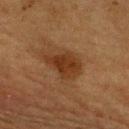<record>
  <biopsy_status>not biopsied; imaged during a skin examination</biopsy_status>
  <automated_metrics>
    <vs_skin_darker_L>8.0</vs_skin_darker_L>
    <vs_skin_contrast_norm>8.5</vs_skin_contrast_norm>
    <nevus_likeness_0_100>50</nevus_likeness_0_100>
  </automated_metrics>
  <patient>
    <sex>male</sex>
    <age_approx>75</age_approx>
  </patient>
  <site>front of the torso</site>
  <image>
    <source>total-body photography crop</source>
    <field_of_view_mm>15</field_of_view_mm>
  </image>
  <lesion_size>
    <long_diameter_mm_approx>4.5</long_diameter_mm_approx>
  </lesion_size>
</record>